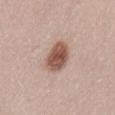A female patient aged 63–67.
The recorded lesion diameter is about 4 mm.
The lesion is located on the lower back.
Automated image analysis of the tile measured a footprint of about 10 mm², an eccentricity of roughly 0.55, and a shape-asymmetry score of about 0.15 (0 = symmetric). The software also gave a lesion-to-skin contrast of about 10 (normalized; higher = more distinct).
A lesion tile, about 15 mm wide, cut from a 3D total-body photograph.
This is a white-light tile.